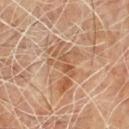Q: Was this lesion biopsied?
A: no biopsy performed (imaged during a skin exam)
Q: Lesion location?
A: the chest
Q: Patient demographics?
A: male, in their 70s
Q: What kind of image is this?
A: total-body-photography crop, ~15 mm field of view